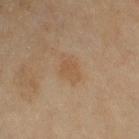The lesion was tiled from a total-body skin photograph and was not biopsied. A close-up tile cropped from a whole-body skin photograph, about 15 mm across. Automated image analysis of the tile measured a border-irregularity index near 3/10, a within-lesion color-variation index near 1.5/10, and radial color variation of about 0.5. The lesion is located on the right upper arm. The patient is a female approximately 60 years of age.